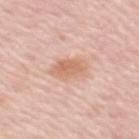- notes — no biopsy performed (imaged during a skin exam)
- image source — total-body-photography crop, ~15 mm field of view
- patient — female, roughly 50 years of age
- site — the arm
- automated lesion analysis — a lesion area of about 8 mm², an outline eccentricity of about 0.8 (0 = round, 1 = elongated), and a symmetry-axis asymmetry near 0.2; an automated nevus-likeness rating near 80 out of 100 and a lesion-detection confidence of about 100/100
- tile lighting — white-light